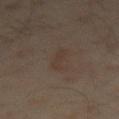{
  "biopsy_status": "not biopsied; imaged during a skin examination",
  "lighting": "cross-polarized",
  "patient": {
    "sex": "male",
    "age_approx": 60
  },
  "site": "left thigh",
  "image": {
    "source": "total-body photography crop",
    "field_of_view_mm": 15
  },
  "automated_metrics": {
    "area_mm2_approx": 3.5,
    "eccentricity": 0.95,
    "shape_asymmetry": 0.35,
    "vs_skin_darker_L": 4.0,
    "vs_skin_contrast_norm": 4.5,
    "color_variation_0_10": 0.0,
    "peripheral_color_asymmetry": 0.0,
    "lesion_detection_confidence_0_100": 100
  },
  "lesion_size": {
    "long_diameter_mm_approx": 4.0
  }
}